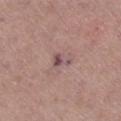| key | value |
|---|---|
| follow-up | catalogued during a skin exam; not biopsied |
| lesion diameter | ≈2.5 mm |
| patient | female, in their 50s |
| acquisition | ~15 mm tile from a whole-body skin photo |
| site | the leg |